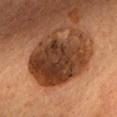{"biopsy_status": "not biopsied; imaged during a skin examination", "lighting": "cross-polarized", "patient": {"sex": "female", "age_approx": 60}, "site": "head or neck", "image": {"source": "total-body photography crop", "field_of_view_mm": 15}}